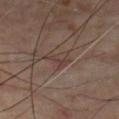| key | value |
|---|---|
| biopsy status | catalogued during a skin exam; not biopsied |